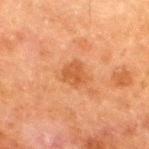patient — male, in their 80s | tile lighting — cross-polarized illumination | location — the right thigh | TBP lesion metrics — an average lesion color of about L≈44 a*≈23 b*≈35 (CIELAB) and a lesion-to-skin contrast of about 6.5 (normalized; higher = more distinct) | lesion diameter — about 3 mm | image — 15 mm crop, total-body photography.